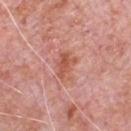{
  "automated_metrics": {
    "cielab_L": 57,
    "cielab_a": 27,
    "cielab_b": 31,
    "vs_skin_darker_L": 8.0,
    "vs_skin_contrast_norm": 6.5,
    "peripheral_color_asymmetry": 1.5
  },
  "lighting": "white-light",
  "lesion_size": {
    "long_diameter_mm_approx": 4.0
  },
  "patient": {
    "sex": "male",
    "age_approx": 80
  },
  "image": {
    "source": "total-body photography crop",
    "field_of_view_mm": 15
  },
  "site": "chest"
}